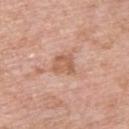  patient:
    sex: male
    age_approx: 60
  lighting: white-light
  lesion_size:
    long_diameter_mm_approx: 2.5
  site: upper back
  image:
    source: total-body photography crop
    field_of_view_mm: 15